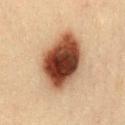follow-up = catalogued during a skin exam; not biopsied | image source = 15 mm crop, total-body photography | patient = male, approximately 35 years of age | body site = the chest.